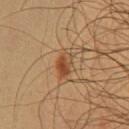Background:
The total-body-photography lesion software estimated a mean CIELAB color near L≈43 a*≈21 b*≈33, a lesion–skin lightness drop of about 10, and a normalized border contrast of about 8. And it measured a nevus-likeness score of about 95/100 and a lesion-detection confidence of about 100/100. Captured under cross-polarized illumination. A male subject, approximately 35 years of age. This image is a 15 mm lesion crop taken from a total-body photograph. Approximately 3 mm at its widest. On the chest.